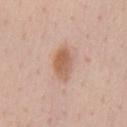Clinical impression:
Part of a total-body skin-imaging series; this lesion was reviewed on a skin check and was not flagged for biopsy.
Image and clinical context:
The recorded lesion diameter is about 4.5 mm. The tile uses white-light illumination. On the chest. The subject is a male aged 53 to 57. A lesion tile, about 15 mm wide, cut from a 3D total-body photograph. The total-body-photography lesion software estimated a lesion area of about 8 mm² and a shape eccentricity near 0.9. The software also gave a border-irregularity index near 2.5/10, a within-lesion color-variation index near 4/10, and a peripheral color-asymmetry measure near 1.5.